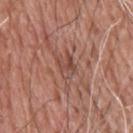Q: Was this lesion biopsied?
A: no biopsy performed (imaged during a skin exam)
Q: Lesion location?
A: the back
Q: Lesion size?
A: about 3 mm
Q: What is the imaging modality?
A: total-body-photography crop, ~15 mm field of view
Q: Who is the patient?
A: male, aged 58 to 62